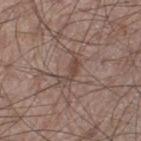biopsy status = imaged on a skin check; not biopsied
subject = male, about 60 years old
lighting = white-light
diameter = ≈4 mm
body site = the left thigh
image = 15 mm crop, total-body photography